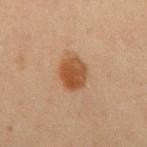Imaged during a routine full-body skin examination; the lesion was not biopsied and no histopathology is available.
The patient is a male aged 58–62.
The tile uses cross-polarized illumination.
From the right upper arm.
About 4 mm across.
A roughly 15 mm field-of-view crop from a total-body skin photograph.
The lesion-visualizer software estimated a lesion color around L≈41 a*≈19 b*≈31 in CIELAB, roughly 10 lightness units darker than nearby skin, and a normalized lesion–skin contrast near 9. The software also gave internal color variation of about 3.5 on a 0–10 scale. The analysis additionally found an automated nevus-likeness rating near 100 out of 100 and a detector confidence of about 100 out of 100 that the crop contains a lesion.